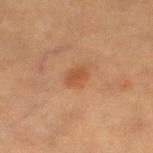<case>
  <biopsy_status>not biopsied; imaged during a skin examination</biopsy_status>
  <patient>
    <sex>female</sex>
    <age_approx>40</age_approx>
  </patient>
  <image>
    <source>total-body photography crop</source>
    <field_of_view_mm>15</field_of_view_mm>
  </image>
  <site>left lower leg</site>
</case>